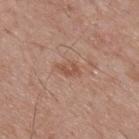Q: Was this lesion biopsied?
A: no biopsy performed (imaged during a skin exam)
Q: What kind of image is this?
A: 15 mm crop, total-body photography
Q: Patient demographics?
A: male, aged 68–72
Q: Automated lesion metrics?
A: a lesion area of about 3 mm², an outline eccentricity of about 0.85 (0 = round, 1 = elongated), and a shape-asymmetry score of about 0.3 (0 = symmetric); an average lesion color of about L≈52 a*≈21 b*≈29 (CIELAB), about 8 CIELAB-L* units darker than the surrounding skin, and a lesion-to-skin contrast of about 6 (normalized; higher = more distinct)
Q: What lighting was used for the tile?
A: white-light
Q: What is the anatomic site?
A: the upper back